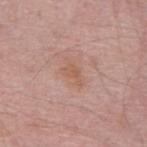workup: no biopsy performed (imaged during a skin exam) | site: the mid back | tile lighting: white-light | lesion size: ~2.5 mm (longest diameter) | patient: male, aged around 60 | image source: ~15 mm tile from a whole-body skin photo.